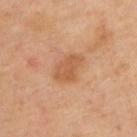Q: Is there a histopathology result?
A: total-body-photography surveillance lesion; no biopsy
Q: Lesion size?
A: ≈3.5 mm
Q: What kind of image is this?
A: 15 mm crop, total-body photography
Q: How was the tile lit?
A: cross-polarized illumination
Q: Who is the patient?
A: female, in their 40s
Q: Where on the body is the lesion?
A: the upper back